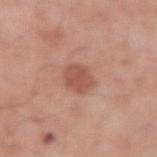Imaged during a routine full-body skin examination; the lesion was not biopsied and no histopathology is available.
Automated tile analysis of the lesion measured a lesion-to-skin contrast of about 7 (normalized; higher = more distinct).
A region of skin cropped from a whole-body photographic capture, roughly 15 mm wide.
A male subject, in their mid-50s.
From the arm.
About 3 mm across.